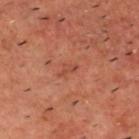Captured during whole-body skin photography for melanoma surveillance; the lesion was not biopsied.
This is a cross-polarized tile.
Cropped from a whole-body photographic skin survey; the tile spans about 15 mm.
The subject is a male in their 60s.
The lesion is located on the head or neck.